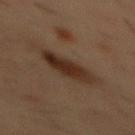Q: Was this lesion biopsied?
A: no biopsy performed (imaged during a skin exam)
Q: What are the patient's age and sex?
A: male, aged 53 to 57
Q: Lesion size?
A: about 5.5 mm
Q: What did automated image analysis measure?
A: a footprint of about 11 mm², an outline eccentricity of about 0.9 (0 = round, 1 = elongated), and a symmetry-axis asymmetry near 0.25; roughly 9 lightness units darker than nearby skin and a normalized border contrast of about 10; a nevus-likeness score of about 55/100 and a lesion-detection confidence of about 100/100
Q: What kind of image is this?
A: ~15 mm crop, total-body skin-cancer survey
Q: Lesion location?
A: the chest